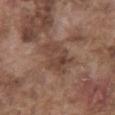Q: What did automated image analysis measure?
A: an outline eccentricity of about 0.8 (0 = round, 1 = elongated); a mean CIELAB color near L≈42 a*≈18 b*≈25, roughly 7 lightness units darker than nearby skin, and a normalized lesion–skin contrast near 6; border irregularity of about 4 on a 0–10 scale, a color-variation rating of about 4/10, and radial color variation of about 1.5; a classifier nevus-likeness of about 0/100 and a detector confidence of about 100 out of 100 that the crop contains a lesion
Q: Patient demographics?
A: male, roughly 75 years of age
Q: What is the anatomic site?
A: the abdomen
Q: How large is the lesion?
A: about 4.5 mm
Q: What is the imaging modality?
A: ~15 mm tile from a whole-body skin photo